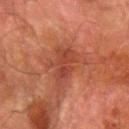Case summary:
- workup · catalogued during a skin exam; not biopsied